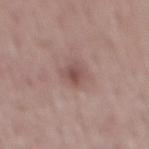Automated tile analysis of the lesion measured roughly 10 lightness units darker than nearby skin and a normalized border contrast of about 7. The software also gave an automated nevus-likeness rating near 5 out of 100 and a lesion-detection confidence of about 100/100. This is a white-light tile. A roughly 15 mm field-of-view crop from a total-body skin photograph. The lesion is located on the mid back. A male subject aged 53–57.